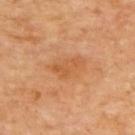The lesion was tiled from a total-body skin photograph and was not biopsied.
The lesion is located on the upper back.
A 15 mm close-up extracted from a 3D total-body photography capture.
Approximately 4.5 mm at its widest.
Imaged with cross-polarized lighting.
The patient is aged 63–67.